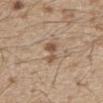Clinical impression:
The lesion was photographed on a routine skin check and not biopsied; there is no pathology result.
Image and clinical context:
Captured under white-light illumination. The patient is a male roughly 70 years of age. Located on the chest. A close-up tile cropped from a whole-body skin photograph, about 15 mm across. The lesion-visualizer software estimated a lesion–skin lightness drop of about 10 and a lesion-to-skin contrast of about 7.5 (normalized; higher = more distinct). It also reported a classifier nevus-likeness of about 10/100 and a lesion-detection confidence of about 100/100.